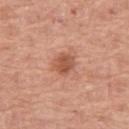This lesion was catalogued during total-body skin photography and was not selected for biopsy. A 15 mm close-up extracted from a 3D total-body photography capture. A female patient roughly 60 years of age. The lesion is on the left thigh. This is a white-light tile. The total-body-photography lesion software estimated a footprint of about 5 mm², a shape eccentricity near 0.35, and a symmetry-axis asymmetry near 0.15. The analysis additionally found a mean CIELAB color near L≈54 a*≈26 b*≈33, about 11 CIELAB-L* units darker than the surrounding skin, and a normalized border contrast of about 7.5. The analysis additionally found lesion-presence confidence of about 100/100.